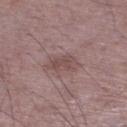follow-up = no biopsy performed (imaged during a skin exam) | image = ~15 mm crop, total-body skin-cancer survey | patient = male, aged 48–52 | TBP lesion metrics = a symmetry-axis asymmetry near 0.3; an average lesion color of about L≈48 a*≈18 b*≈18 (CIELAB), about 8 CIELAB-L* units darker than the surrounding skin, and a normalized border contrast of about 6; a border-irregularity rating of about 3.5/10 and internal color variation of about 2.5 on a 0–10 scale | body site = the right lower leg | size = ≈3.5 mm.